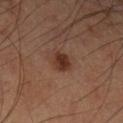<case>
<lighting>cross-polarized</lighting>
<patient>
  <sex>male</sex>
  <age_approx>60</age_approx>
</patient>
<lesion_size>
  <long_diameter_mm_approx>2.5</long_diameter_mm_approx>
</lesion_size>
<image>
  <source>total-body photography crop</source>
  <field_of_view_mm>15</field_of_view_mm>
</image>
<site>left lower leg</site>
</case>